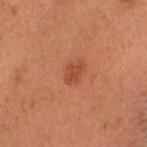Impression:
No biopsy was performed on this lesion — it was imaged during a full skin examination and was not determined to be concerning.